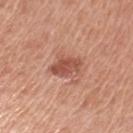This lesion was catalogued during total-body skin photography and was not selected for biopsy. Located on the left upper arm. This is a white-light tile. Cropped from a whole-body photographic skin survey; the tile spans about 15 mm. Automated tile analysis of the lesion measured an average lesion color of about L≈53 a*≈26 b*≈30 (CIELAB), roughly 11 lightness units darker than nearby skin, and a normalized lesion–skin contrast near 7.5. It also reported a nevus-likeness score of about 60/100. A female patient, approximately 55 years of age.